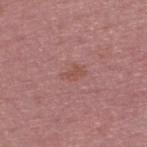site = the upper back
tile lighting = white-light
subject = male, roughly 55 years of age
imaging modality = ~15 mm crop, total-body skin-cancer survey